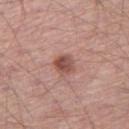The tile uses white-light illumination. From the right thigh. An algorithmic analysis of the crop reported a footprint of about 5.5 mm² and a shape-asymmetry score of about 0.2 (0 = symmetric). It also reported radial color variation of about 1.5. About 2.5 mm across. A male patient, about 55 years old. A lesion tile, about 15 mm wide, cut from a 3D total-body photograph.